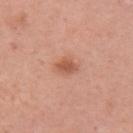| feature | finding |
|---|---|
| biopsy status | catalogued during a skin exam; not biopsied |
| size | ≈3 mm |
| tile lighting | white-light |
| TBP lesion metrics | a border-irregularity index near 2/10 and internal color variation of about 2.5 on a 0–10 scale |
| location | the right upper arm |
| imaging modality | ~15 mm tile from a whole-body skin photo |
| patient | female, aged 33–37 |